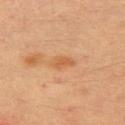follow-up: total-body-photography surveillance lesion; no biopsy | body site: the arm | lesion diameter: ≈3 mm | patient: male, roughly 35 years of age | image: total-body-photography crop, ~15 mm field of view | automated lesion analysis: a lesion–skin lightness drop of about 7 and a normalized border contrast of about 6 | illumination: cross-polarized.